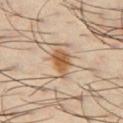Assessment: Recorded during total-body skin imaging; not selected for excision or biopsy. Clinical summary: Located on the chest. The tile uses cross-polarized illumination. Measured at roughly 3.5 mm in maximum diameter. Automated image analysis of the tile measured a footprint of about 6.5 mm², an eccentricity of roughly 0.75, and two-axis asymmetry of about 0.3. And it measured an average lesion color of about L≈55 a*≈19 b*≈35 (CIELAB) and a normalized lesion–skin contrast near 9.5. A close-up tile cropped from a whole-body skin photograph, about 15 mm across. A male subject, approximately 40 years of age.